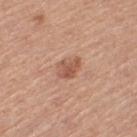| feature | finding |
|---|---|
| notes | no biopsy performed (imaged during a skin exam) |
| automated lesion analysis | a mean CIELAB color near L≈55 a*≈23 b*≈31; a border-irregularity index near 3/10, a within-lesion color-variation index near 3/10, and peripheral color asymmetry of about 1; an automated nevus-likeness rating near 35 out of 100 and a lesion-detection confidence of about 100/100 |
| illumination | white-light |
| subject | female, in their mid-50s |
| body site | the left thigh |
| lesion size | ≈3 mm |
| image source | ~15 mm crop, total-body skin-cancer survey |